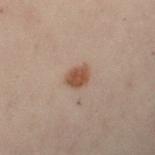Recorded during total-body skin imaging; not selected for excision or biopsy. Approximately 2.5 mm at its widest. The total-body-photography lesion software estimated an average lesion color of about L≈39 a*≈16 b*≈24 (CIELAB), a lesion–skin lightness drop of about 10, and a lesion-to-skin contrast of about 9 (normalized; higher = more distinct). And it measured a border-irregularity rating of about 1.5/10, a color-variation rating of about 2/10, and a peripheral color-asymmetry measure near 0.5. The analysis additionally found a classifier nevus-likeness of about 100/100 and lesion-presence confidence of about 100/100. The lesion is located on the left forearm. A region of skin cropped from a whole-body photographic capture, roughly 15 mm wide. Imaged with cross-polarized lighting. A female patient aged 28 to 32.